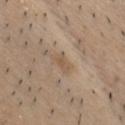workup — catalogued during a skin exam; not biopsied | subject — male, aged 33–37 | image — 15 mm crop, total-body photography | location — the head or neck | tile lighting — white-light | image-analysis metrics — an area of roughly 3.5 mm² and two-axis asymmetry of about 0.35; a classifier nevus-likeness of about 0/100 and lesion-presence confidence of about 100/100 | lesion diameter — ≈2.5 mm.